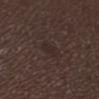Clinical impression:
The lesion was photographed on a routine skin check and not biopsied; there is no pathology result.
Image and clinical context:
The patient is a female aged approximately 50. Captured under white-light illumination. Approximately 2.5 mm at its widest. The lesion is located on the left lower leg. A lesion tile, about 15 mm wide, cut from a 3D total-body photograph.